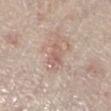Clinical impression: Recorded during total-body skin imaging; not selected for excision or biopsy. Background: The recorded lesion diameter is about 3 mm. Imaged with white-light lighting. The lesion is located on the leg. A female patient, approximately 70 years of age. A 15 mm close-up tile from a total-body photography series done for melanoma screening.